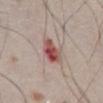This lesion was catalogued during total-body skin photography and was not selected for biopsy. A male patient in their 80s. On the abdomen. A 15 mm close-up extracted from a 3D total-body photography capture.